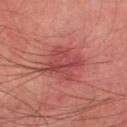The lesion was photographed on a routine skin check and not biopsied; there is no pathology result. A roughly 15 mm field-of-view crop from a total-body skin photograph. A male patient, aged approximately 75. Imaged with cross-polarized lighting. The lesion is on the left thigh. Measured at roughly 4.5 mm in maximum diameter. Automated tile analysis of the lesion measured a lesion area of about 14 mm². The analysis additionally found an average lesion color of about L≈39 a*≈28 b*≈22 (CIELAB), about 8 CIELAB-L* units darker than the surrounding skin, and a lesion-to-skin contrast of about 6.5 (normalized; higher = more distinct).